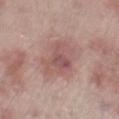No biopsy was performed on this lesion — it was imaged during a full skin examination and was not determined to be concerning. From the right thigh. Cropped from a total-body skin-imaging series; the visible field is about 15 mm. A male subject, in their mid- to late 60s.